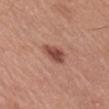Part of a total-body skin-imaging series; this lesion was reviewed on a skin check and was not flagged for biopsy. The lesion-visualizer software estimated an average lesion color of about L≈48 a*≈25 b*≈27 (CIELAB), roughly 13 lightness units darker than nearby skin, and a normalized border contrast of about 9. Located on the right upper arm. A male patient, aged 53 to 57. Approximately 3 mm at its widest. This is a white-light tile. A close-up tile cropped from a whole-body skin photograph, about 15 mm across.